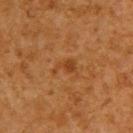biopsy status = no biopsy performed (imaged during a skin exam); illumination = cross-polarized; patient = male, approximately 60 years of age; body site = the upper back; acquisition = ~15 mm tile from a whole-body skin photo; lesion size = ~3 mm (longest diameter).